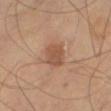{"biopsy_status": "not biopsied; imaged during a skin examination", "site": "left leg", "patient": {"sex": "male", "age_approx": 65}, "lighting": "cross-polarized", "image": {"source": "total-body photography crop", "field_of_view_mm": 15}, "automated_metrics": {"area_mm2_approx": 7.0, "eccentricity": 0.65, "shape_asymmetry": 0.15, "nevus_likeness_0_100": 75, "lesion_detection_confidence_0_100": 100}}